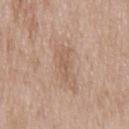Notes:
* follow-up: total-body-photography surveillance lesion; no biopsy
* lesion diameter: about 3.5 mm
* tile lighting: white-light illumination
* image source: total-body-photography crop, ~15 mm field of view
* patient: male, aged around 50
* automated lesion analysis: a shape-asymmetry score of about 0.45 (0 = symmetric); a mean CIELAB color near L≈58 a*≈18 b*≈29, a lesion–skin lightness drop of about 7, and a normalized border contrast of about 5; a classifier nevus-likeness of about 0/100 and lesion-presence confidence of about 100/100
* body site: the chest